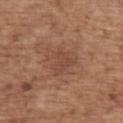<tbp_lesion>
<biopsy_status>not biopsied; imaged during a skin examination</biopsy_status>
<lesion_size>
  <long_diameter_mm_approx>4.0</long_diameter_mm_approx>
</lesion_size>
<lighting>white-light</lighting>
<automated_metrics>
  <area_mm2_approx>9.0</area_mm2_approx>
  <eccentricity>0.7</eccentricity>
  <shape_asymmetry>0.3</shape_asymmetry>
  <border_irregularity_0_10>3.5</border_irregularity_0_10>
  <color_variation_0_10>2.0</color_variation_0_10>
</automated_metrics>
<image>
  <source>total-body photography crop</source>
  <field_of_view_mm>15</field_of_view_mm>
</image>
<site>upper back</site>
<patient>
  <sex>male</sex>
  <age_approx>70</age_approx>
</patient>
</tbp_lesion>